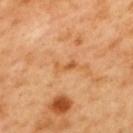| field | value |
|---|---|
| notes | catalogued during a skin exam; not biopsied |
| location | the mid back |
| patient | male, aged around 50 |
| diameter | ≈3 mm |
| acquisition | total-body-photography crop, ~15 mm field of view |